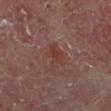The patient is a male aged approximately 60. The lesion is located on the left lower leg. A 15 mm close-up extracted from a 3D total-body photography capture.